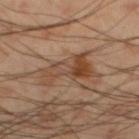| key | value |
|---|---|
| workup | no biopsy performed (imaged during a skin exam) |
| subject | male, aged 53–57 |
| lesion size | ≈5.5 mm |
| location | the leg |
| imaging modality | 15 mm crop, total-body photography |
| tile lighting | cross-polarized |